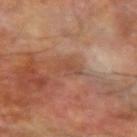Clinical impression: The lesion was tiled from a total-body skin photograph and was not biopsied. Background: From the left forearm. An algorithmic analysis of the crop reported a lesion area of about 3 mm², an outline eccentricity of about 0.75 (0 = round, 1 = elongated), and a shape-asymmetry score of about 0.45 (0 = symmetric). The software also gave a mean CIELAB color near L≈46 a*≈23 b*≈29, a lesion–skin lightness drop of about 6, and a normalized lesion–skin contrast near 5. The tile uses cross-polarized illumination. The lesion's longest dimension is about 2.5 mm. A male patient aged 68–72. A 15 mm close-up tile from a total-body photography series done for melanoma screening.